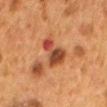Part of a total-body skin-imaging series; this lesion was reviewed on a skin check and was not flagged for biopsy.
This image is a 15 mm lesion crop taken from a total-body photograph.
A female subject, approximately 50 years of age.
The lesion is located on the mid back.
Imaged with cross-polarized lighting.
The lesion's longest dimension is about 4 mm.